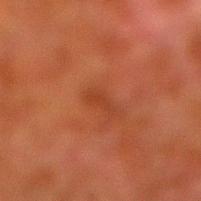The lesion was tiled from a total-body skin photograph and was not biopsied.
A male subject roughly 80 years of age.
A roughly 15 mm field-of-view crop from a total-body skin photograph.
The lesion's longest dimension is about 2.5 mm.
The lesion is located on the right lower leg.
Imaged with cross-polarized lighting.